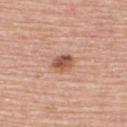  biopsy_status: not biopsied; imaged during a skin examination
  site: upper back
  patient:
    sex: female
    age_approx: 60
  image:
    source: total-body photography crop
    field_of_view_mm: 15
  automated_metrics:
    cielab_L: 55
    cielab_a: 23
    cielab_b: 31
    vs_skin_darker_L: 13.0
    vs_skin_contrast_norm: 8.5
    border_irregularity_0_10: 1.0
    color_variation_0_10: 5.0
    nevus_likeness_0_100: 95
    lesion_detection_confidence_0_100: 100
  lesion_size:
    long_diameter_mm_approx: 3.0
  lighting: white-light